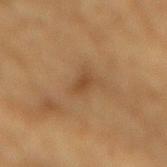Recorded during total-body skin imaging; not selected for excision or biopsy. The patient is a male roughly 85 years of age or older. From the mid back. A roughly 15 mm field-of-view crop from a total-body skin photograph.